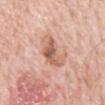Q: Who is the patient?
A: male, approximately 80 years of age
Q: What is the imaging modality?
A: 15 mm crop, total-body photography
Q: Where on the body is the lesion?
A: the mid back
Q: Illumination type?
A: white-light illumination
Q: Lesion size?
A: ≈6 mm